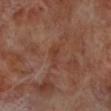  biopsy_status: not biopsied; imaged during a skin examination
  image:
    source: total-body photography crop
    field_of_view_mm: 15
  patient:
    sex: male
    age_approx: 70
  lighting: cross-polarized
  site: leg
  lesion_size:
    long_diameter_mm_approx: 3.5